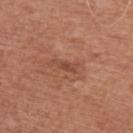Imaged during a routine full-body skin examination; the lesion was not biopsied and no histopathology is available. On the left upper arm. This is a white-light tile. A male subject, approximately 65 years of age. About 3 mm across. This image is a 15 mm lesion crop taken from a total-body photograph. The total-body-photography lesion software estimated a lesion area of about 3 mm², a shape eccentricity near 0.9, and a symmetry-axis asymmetry near 0.4.